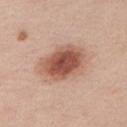Case summary:
- automated metrics: border irregularity of about 2 on a 0–10 scale, a color-variation rating of about 7/10, and a peripheral color-asymmetry measure near 1.5
- acquisition: total-body-photography crop, ~15 mm field of view
- body site: the chest
- subject: female, aged approximately 45
- illumination: white-light